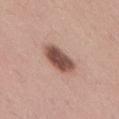Captured during whole-body skin photography for melanoma surveillance; the lesion was not biopsied. Captured under white-light illumination. The patient is a male roughly 30 years of age. Approximately 4.5 mm at its widest. From the lower back. This image is a 15 mm lesion crop taken from a total-body photograph.